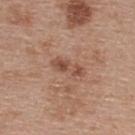{"biopsy_status": "not biopsied; imaged during a skin examination", "lesion_size": {"long_diameter_mm_approx": 4.0}, "patient": {"sex": "female", "age_approx": 40}, "site": "back", "image": {"source": "total-body photography crop", "field_of_view_mm": 15}, "lighting": "white-light"}